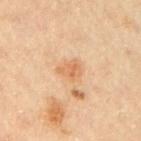biopsy status — imaged on a skin check; not biopsied | lighting — cross-polarized illumination | lesion size — ≈2.5 mm | site — the right upper arm | patient — male, in their 60s | imaging modality — total-body-photography crop, ~15 mm field of view.